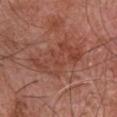biopsy status — imaged on a skin check; not biopsied
patient — male, aged around 65
body site — the chest
image — ~15 mm crop, total-body skin-cancer survey